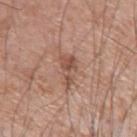Imaged during a routine full-body skin examination; the lesion was not biopsied and no histopathology is available.
Cropped from a whole-body photographic skin survey; the tile spans about 15 mm.
Located on the left upper arm.
A male subject, in their mid- to late 70s.